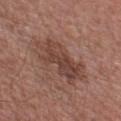Q: Was this lesion biopsied?
A: total-body-photography surveillance lesion; no biopsy
Q: Illumination type?
A: white-light illumination
Q: What is the imaging modality?
A: ~15 mm crop, total-body skin-cancer survey
Q: What is the lesion's diameter?
A: ≈7.5 mm
Q: Automated lesion metrics?
A: a border-irregularity rating of about 6/10, a color-variation rating of about 4.5/10, and a peripheral color-asymmetry measure near 1.5
Q: Lesion location?
A: the chest
Q: What are the patient's age and sex?
A: male, aged 63 to 67